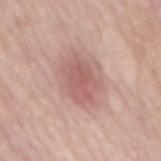Context: Imaged with white-light lighting. Approximately 5 mm at its widest. Located on the mid back. Automated tile analysis of the lesion measured a peripheral color-asymmetry measure near 1. The software also gave an automated nevus-likeness rating near 40 out of 100. A roughly 15 mm field-of-view crop from a total-body skin photograph. The subject is a female in their mid- to late 60s.